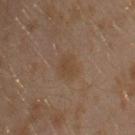Impression:
Part of a total-body skin-imaging series; this lesion was reviewed on a skin check and was not flagged for biopsy.
Background:
The lesion is on the left upper arm. The total-body-photography lesion software estimated a lesion–skin lightness drop of about 5 and a normalized border contrast of about 5.5. It also reported border irregularity of about 2.5 on a 0–10 scale, internal color variation of about 1.5 on a 0–10 scale, and radial color variation of about 0.5. Imaged with cross-polarized lighting. A male subject about 30 years old. A lesion tile, about 15 mm wide, cut from a 3D total-body photograph.